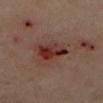{
  "site": "left lower leg",
  "image": {
    "source": "total-body photography crop",
    "field_of_view_mm": 15
  },
  "patient": {
    "sex": "male",
    "age_approx": 65
  }
}